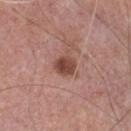notes=catalogued during a skin exam; not biopsied
location=the chest
acquisition=15 mm crop, total-body photography
automated lesion analysis=border irregularity of about 1.5 on a 0–10 scale, internal color variation of about 3.5 on a 0–10 scale, and radial color variation of about 1.5
patient=male, roughly 75 years of age
diameter=~2.5 mm (longest diameter)